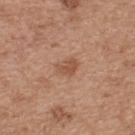Q: Was a biopsy performed?
A: total-body-photography surveillance lesion; no biopsy
Q: Lesion location?
A: the upper back
Q: How was this image acquired?
A: 15 mm crop, total-body photography
Q: What lighting was used for the tile?
A: white-light
Q: Who is the patient?
A: female, roughly 40 years of age
Q: Lesion size?
A: ~2.5 mm (longest diameter)